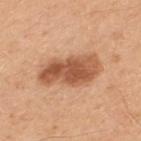Findings:
• notes — total-body-photography surveillance lesion; no biopsy
• diameter — ~7 mm (longest diameter)
• subject — male, approximately 50 years of age
• image source — ~15 mm tile from a whole-body skin photo
• tile lighting — white-light
• image-analysis metrics — an area of roughly 18 mm² and a shape eccentricity near 0.9; a border-irregularity index near 3/10 and radial color variation of about 2; a classifier nevus-likeness of about 85/100 and lesion-presence confidence of about 100/100
• body site — the upper back